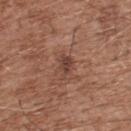– biopsy status · imaged on a skin check; not biopsied
– image · ~15 mm tile from a whole-body skin photo
– tile lighting · white-light
– site · the upper back
– lesion diameter · about 2.5 mm
– TBP lesion metrics · about 9 CIELAB-L* units darker than the surrounding skin and a normalized border contrast of about 7
– subject · male, about 75 years old